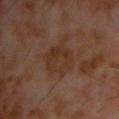Case summary:
* notes: imaged on a skin check; not biopsied
* diameter: about 4.5 mm
* tile lighting: cross-polarized illumination
* imaging modality: ~15 mm crop, total-body skin-cancer survey
* automated lesion analysis: a lesion area of about 11 mm², an eccentricity of roughly 0.75, and a shape-asymmetry score of about 0.2 (0 = symmetric); about 5 CIELAB-L* units darker than the surrounding skin and a normalized border contrast of about 6.5; a border-irregularity index near 2.5/10 and internal color variation of about 3.5 on a 0–10 scale; a classifier nevus-likeness of about 0/100 and a detector confidence of about 100 out of 100 that the crop contains a lesion
* site: the chest
* subject: male, aged around 60